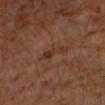* workup · imaged on a skin check; not biopsied
* lesion diameter · ≈4 mm
* acquisition · ~15 mm crop, total-body skin-cancer survey
* automated lesion analysis · an outline eccentricity of about 0.95 (0 = round, 1 = elongated) and two-axis asymmetry of about 0.45; a mean CIELAB color near L≈31 a*≈18 b*≈27, a lesion–skin lightness drop of about 6, and a normalized lesion–skin contrast near 6; a border-irregularity rating of about 6/10, a within-lesion color-variation index near 1/10, and peripheral color asymmetry of about 0
* patient · male, in their 60s
* anatomic site · the left upper arm
* illumination · cross-polarized